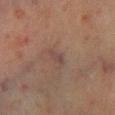{
  "biopsy_status": "not biopsied; imaged during a skin examination",
  "image": {
    "source": "total-body photography crop",
    "field_of_view_mm": 15
  },
  "site": "left lower leg",
  "patient": {
    "sex": "male",
    "age_approx": 70
  }
}